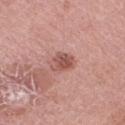Q: Was this lesion biopsied?
A: no biopsy performed (imaged during a skin exam)
Q: Illumination type?
A: white-light
Q: What is the imaging modality?
A: ~15 mm tile from a whole-body skin photo
Q: Lesion size?
A: ≈3 mm
Q: Where on the body is the lesion?
A: the leg
Q: Automated lesion metrics?
A: a lesion area of about 5 mm², an eccentricity of roughly 0.7, and two-axis asymmetry of about 0.3; a mean CIELAB color near L≈53 a*≈25 b*≈25, a lesion–skin lightness drop of about 12, and a normalized lesion–skin contrast near 8
Q: What are the patient's age and sex?
A: female, about 70 years old